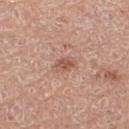Captured during whole-body skin photography for melanoma surveillance; the lesion was not biopsied.
The lesion is on the left thigh.
The patient is a female in their 50s.
The lesion-visualizer software estimated an area of roughly 4.5 mm², an eccentricity of roughly 0.75, and a symmetry-axis asymmetry near 0.15. The software also gave a within-lesion color-variation index near 4/10 and peripheral color asymmetry of about 1.5. The software also gave a lesion-detection confidence of about 100/100.
Imaged with white-light lighting.
This image is a 15 mm lesion crop taken from a total-body photograph.